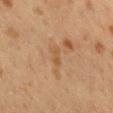notes — catalogued during a skin exam; not biopsied
lesion diameter — ≈2.5 mm
image source — total-body-photography crop, ~15 mm field of view
location — the upper back
subject — female, in their 40s
lighting — cross-polarized illumination